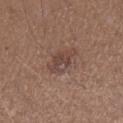Impression: This lesion was catalogued during total-body skin photography and was not selected for biopsy. Clinical summary: Cropped from a total-body skin-imaging series; the visible field is about 15 mm. A female patient, aged around 45. Located on the right forearm. Longest diameter approximately 3.5 mm. The tile uses white-light illumination.